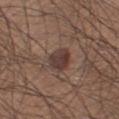Recorded during total-body skin imaging; not selected for excision or biopsy.
Located on the right thigh.
A roughly 15 mm field-of-view crop from a total-body skin photograph.
The lesion-visualizer software estimated a footprint of about 6.5 mm², a shape eccentricity near 0.8, and a symmetry-axis asymmetry near 0.15. And it measured border irregularity of about 1.5 on a 0–10 scale, internal color variation of about 4 on a 0–10 scale, and radial color variation of about 1.5.
A male patient, approximately 55 years of age.
Captured under white-light illumination.
The lesion's longest dimension is about 4 mm.